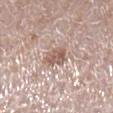Notes:
• biopsy status — no biopsy performed (imaged during a skin exam)
• location — the right lower leg
• subject — female, aged 48–52
• image source — total-body-photography crop, ~15 mm field of view